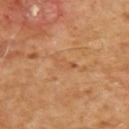The tile uses cross-polarized illumination.
Cropped from a total-body skin-imaging series; the visible field is about 15 mm.
The patient is a male in their mid- to late 50s.
Automated tile analysis of the lesion measured a shape eccentricity near 0.9 and two-axis asymmetry of about 0.4. The software also gave a classifier nevus-likeness of about 0/100.
Located on the upper back.
The biopsy diagnosis was an invasive squamous cell carcinoma.